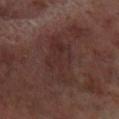This lesion was catalogued during total-body skin photography and was not selected for biopsy. A male patient approximately 70 years of age. A lesion tile, about 15 mm wide, cut from a 3D total-body photograph. Automated image analysis of the tile measured a lesion area of about 16 mm², an outline eccentricity of about 0.85 (0 = round, 1 = elongated), and a shape-asymmetry score of about 0.35 (0 = symmetric). And it measured lesion-presence confidence of about 95/100. The lesion is on the left lower leg. Longest diameter approximately 6.5 mm.